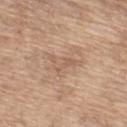• follow-up — no biopsy performed (imaged during a skin exam)